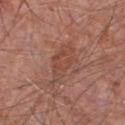Notes:
* biopsy status — imaged on a skin check; not biopsied
* lesion size — ≈5 mm
* anatomic site — the front of the torso
* imaging modality — 15 mm crop, total-body photography
* image-analysis metrics — a border-irregularity index near 4/10 and peripheral color asymmetry of about 1; an automated nevus-likeness rating near 0 out of 100 and a detector confidence of about 95 out of 100 that the crop contains a lesion
* subject — male, approximately 65 years of age